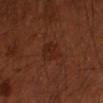This lesion was catalogued during total-body skin photography and was not selected for biopsy. Automated tile analysis of the lesion measured an area of roughly 6.5 mm², an outline eccentricity of about 0.75 (0 = round, 1 = elongated), and two-axis asymmetry of about 0.25. The analysis additionally found a border-irregularity index near 2.5/10, a color-variation rating of about 2/10, and radial color variation of about 1. It also reported an automated nevus-likeness rating near 5 out of 100 and a lesion-detection confidence of about 100/100. Measured at roughly 3.5 mm in maximum diameter. Captured under cross-polarized illumination. From the left forearm. A 15 mm close-up extracted from a 3D total-body photography capture. The patient is a male aged 48 to 52.